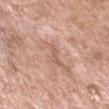workup: catalogued during a skin exam; not biopsied
lighting: white-light
site: the right forearm
lesion diameter: ~3 mm (longest diameter)
subject: female, in their mid-70s
image: ~15 mm tile from a whole-body skin photo